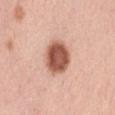| feature | finding |
|---|---|
| workup | imaged on a skin check; not biopsied |
| site | the left thigh |
| imaging modality | 15 mm crop, total-body photography |
| lesion size | about 4 mm |
| illumination | white-light |
| patient | female, aged 43–47 |